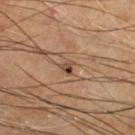<tbp_lesion>
  <biopsy_status>not biopsied; imaged during a skin examination</biopsy_status>
  <automated_metrics>
    <area_mm2_approx>2.0</area_mm2_approx>
    <eccentricity>0.55</eccentricity>
    <shape_asymmetry>0.5</shape_asymmetry>
    <nevus_likeness_0_100>75</nevus_likeness_0_100>
  </automated_metrics>
  <lesion_size>
    <long_diameter_mm_approx>2.0</long_diameter_mm_approx>
  </lesion_size>
  <patient>
    <sex>male</sex>
    <age_approx>70</age_approx>
  </patient>
  <site>right lower leg</site>
  <image>
    <source>total-body photography crop</source>
    <field_of_view_mm>15</field_of_view_mm>
  </image>
  <lighting>cross-polarized</lighting>
</tbp_lesion>